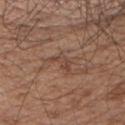Assessment: The lesion was photographed on a routine skin check and not biopsied; there is no pathology result. Image and clinical context: The lesion is located on the left upper arm. A male subject, aged approximately 55. Automated tile analysis of the lesion measured an area of roughly 3.5 mm². The analysis additionally found border irregularity of about 7.5 on a 0–10 scale, internal color variation of about 0 on a 0–10 scale, and peripheral color asymmetry of about 0. A 15 mm crop from a total-body photograph taken for skin-cancer surveillance.